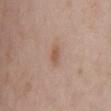The lesion was tiled from a total-body skin photograph and was not biopsied. A 15 mm close-up extracted from a 3D total-body photography capture. From the mid back. The tile uses white-light illumination. The subject is a male approximately 65 years of age. An algorithmic analysis of the crop reported a within-lesion color-variation index near 0.5/10 and peripheral color asymmetry of about 0. It also reported a nevus-likeness score of about 35/100 and a detector confidence of about 100 out of 100 that the crop contains a lesion.